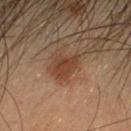Q: Is there a histopathology result?
A: no biopsy performed (imaged during a skin exam)
Q: Who is the patient?
A: male, in their mid- to late 30s
Q: Where on the body is the lesion?
A: the head or neck
Q: How was the tile lit?
A: cross-polarized
Q: What is the lesion's diameter?
A: ≈3.5 mm
Q: Automated lesion metrics?
A: an area of roughly 5 mm²; a lesion color around L≈35 a*≈19 b*≈28 in CIELAB and roughly 8 lightness units darker than nearby skin; border irregularity of about 3 on a 0–10 scale, a color-variation rating of about 1.5/10, and peripheral color asymmetry of about 0.5; a detector confidence of about 100 out of 100 that the crop contains a lesion
Q: What kind of image is this?
A: ~15 mm crop, total-body skin-cancer survey